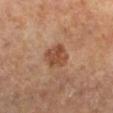workup: total-body-photography surveillance lesion; no biopsy | acquisition: ~15 mm tile from a whole-body skin photo | lesion diameter: about 3 mm | subject: female, aged 68–72 | tile lighting: cross-polarized | site: the right lower leg.